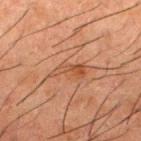This lesion was catalogued during total-body skin photography and was not selected for biopsy. A close-up tile cropped from a whole-body skin photograph, about 15 mm across. The lesion is located on the upper back. This is a cross-polarized tile. Measured at roughly 4 mm in maximum diameter. A male patient, aged approximately 50.